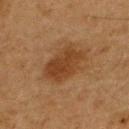{
  "biopsy_status": "not biopsied; imaged during a skin examination",
  "patient": {
    "sex": "male",
    "age_approx": 65
  },
  "automated_metrics": {
    "cielab_L": 34,
    "cielab_a": 18,
    "cielab_b": 30,
    "vs_skin_darker_L": 8.0,
    "vs_skin_contrast_norm": 7.5,
    "peripheral_color_asymmetry": 1.0,
    "nevus_likeness_0_100": 85,
    "lesion_detection_confidence_0_100": 100
  },
  "image": {
    "source": "total-body photography crop",
    "field_of_view_mm": 15
  },
  "lesion_size": {
    "long_diameter_mm_approx": 5.0
  },
  "site": "upper back"
}